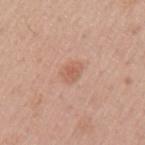The lesion was tiled from a total-body skin photograph and was not biopsied. A lesion tile, about 15 mm wide, cut from a 3D total-body photograph. A male patient, approximately 45 years of age. Approximately 2.5 mm at its widest. The lesion is located on the arm. Automated image analysis of the tile measured an outline eccentricity of about 0.75 (0 = round, 1 = elongated) and two-axis asymmetry of about 0.15. This is a white-light tile.